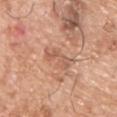<case>
  <biopsy_status>not biopsied; imaged during a skin examination</biopsy_status>
  <lesion_size>
    <long_diameter_mm_approx>4.5</long_diameter_mm_approx>
  </lesion_size>
  <patient>
    <sex>male</sex>
    <age_approx>75</age_approx>
  </patient>
  <site>front of the torso</site>
  <automated_metrics>
    <area_mm2_approx>5.5</area_mm2_approx>
    <cielab_L>58</cielab_L>
    <cielab_a>22</cielab_a>
    <cielab_b>32</cielab_b>
    <vs_skin_darker_L>8.0</vs_skin_darker_L>
    <vs_skin_contrast_norm>5.5</vs_skin_contrast_norm>
  </automated_metrics>
  <lighting>white-light</lighting>
  <image>
    <source>total-body photography crop</source>
    <field_of_view_mm>15</field_of_view_mm>
  </image>
</case>